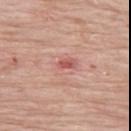automated_metrics:
  nevus_likeness_0_100: 0
  lesion_detection_confidence_0_100: 100
site: upper back
patient:
  sex: female
  age_approx: 70
image:
  source: total-body photography crop
  field_of_view_mm: 15
lighting: white-light